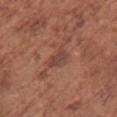Q: Was a biopsy performed?
A: imaged on a skin check; not biopsied
Q: What is the anatomic site?
A: the upper back
Q: What are the patient's age and sex?
A: female, aged 73–77
Q: How was this image acquired?
A: ~15 mm crop, total-body skin-cancer survey